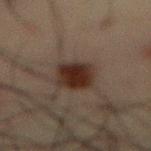Assessment:
No biopsy was performed on this lesion — it was imaged during a full skin examination and was not determined to be concerning.
Clinical summary:
A male patient, aged approximately 60. Imaged with cross-polarized lighting. From the abdomen. The recorded lesion diameter is about 3.5 mm. A close-up tile cropped from a whole-body skin photograph, about 15 mm across.